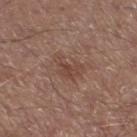Clinical impression:
Captured during whole-body skin photography for melanoma surveillance; the lesion was not biopsied.
Context:
Cropped from a total-body skin-imaging series; the visible field is about 15 mm. The lesion is on the leg. The subject is a male aged around 55. The lesion-visualizer software estimated an outline eccentricity of about 0.8 (0 = round, 1 = elongated) and a symmetry-axis asymmetry near 0.5. And it measured a nevus-likeness score of about 0/100 and lesion-presence confidence of about 100/100.